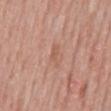<tbp_lesion>
  <biopsy_status>not biopsied; imaged during a skin examination</biopsy_status>
  <site>back</site>
  <patient>
    <sex>male</sex>
    <age_approx>50</age_approx>
  </patient>
  <image>
    <source>total-body photography crop</source>
    <field_of_view_mm>15</field_of_view_mm>
  </image>
</tbp_lesion>